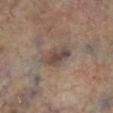Notes:
– notes: catalogued during a skin exam; not biopsied
– site: the left lower leg
– image: ~15 mm crop, total-body skin-cancer survey
– size: ≈4.5 mm
– lighting: cross-polarized illumination
– subject: female, aged approximately 60